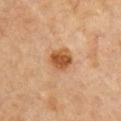biopsy status: total-body-photography surveillance lesion; no biopsy
automated metrics: a symmetry-axis asymmetry near 0.15; a lesion color around L≈52 a*≈23 b*≈39 in CIELAB and a lesion-to-skin contrast of about 9.5 (normalized; higher = more distinct); a border-irregularity rating of about 1.5/10, a within-lesion color-variation index near 4/10, and radial color variation of about 1; a lesion-detection confidence of about 100/100
diameter: about 3 mm
body site: the front of the torso
subject: female, approximately 65 years of age
imaging modality: 15 mm crop, total-body photography